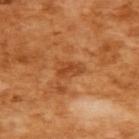Impression:
Imaged during a routine full-body skin examination; the lesion was not biopsied and no histopathology is available.
Background:
Approximately 3 mm at its widest. An algorithmic analysis of the crop reported a lesion color around L≈48 a*≈28 b*≈43 in CIELAB and about 8 CIELAB-L* units darker than the surrounding skin. It also reported an automated nevus-likeness rating near 0 out of 100 and lesion-presence confidence of about 100/100. A female patient, roughly 55 years of age. A lesion tile, about 15 mm wide, cut from a 3D total-body photograph. The lesion is located on the upper back.